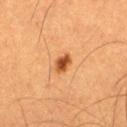Q: Was this lesion biopsied?
A: imaged on a skin check; not biopsied
Q: What did automated image analysis measure?
A: an area of roughly 3.5 mm², an outline eccentricity of about 0.75 (0 = round, 1 = elongated), and two-axis asymmetry of about 0.3; a lesion color around L≈49 a*≈28 b*≈41 in CIELAB, roughly 15 lightness units darker than nearby skin, and a normalized border contrast of about 10.5
Q: Where on the body is the lesion?
A: the right thigh
Q: What is the imaging modality?
A: total-body-photography crop, ~15 mm field of view
Q: Lesion size?
A: about 2.5 mm
Q: What are the patient's age and sex?
A: male, aged 58–62